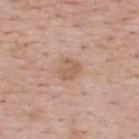Imaged during a routine full-body skin examination; the lesion was not biopsied and no histopathology is available.
Located on the back.
Imaged with white-light lighting.
Measured at roughly 2.5 mm in maximum diameter.
The patient is a male aged approximately 55.
An algorithmic analysis of the crop reported border irregularity of about 3 on a 0–10 scale and a peripheral color-asymmetry measure near 0.5. And it measured an automated nevus-likeness rating near 0 out of 100 and lesion-presence confidence of about 100/100.
This image is a 15 mm lesion crop taken from a total-body photograph.